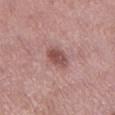The lesion-visualizer software estimated a lesion area of about 5.5 mm² and a shape-asymmetry score of about 0.15 (0 = symmetric). It also reported internal color variation of about 3 on a 0–10 scale and peripheral color asymmetry of about 1. A female patient, about 50 years old. From the left lower leg. This image is a 15 mm lesion crop taken from a total-body photograph. Captured under white-light illumination. Measured at roughly 3 mm in maximum diameter.